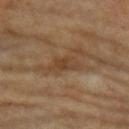Assessment: Part of a total-body skin-imaging series; this lesion was reviewed on a skin check and was not flagged for biopsy. Background: Captured under cross-polarized illumination. On the left upper arm. A roughly 15 mm field-of-view crop from a total-body skin photograph. Measured at roughly 3.5 mm in maximum diameter. The subject is a female in their mid-60s.